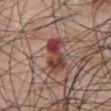TBP lesion metrics: a lesion color around L≈43 a*≈24 b*≈24 in CIELAB, about 13 CIELAB-L* units darker than the surrounding skin, and a normalized border contrast of about 10
illumination: white-light
site: the chest
patient: male, about 70 years old
lesion diameter: ≈4.5 mm
image: ~15 mm tile from a whole-body skin photo